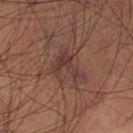Captured during whole-body skin photography for melanoma surveillance; the lesion was not biopsied. The lesion is on the left lower leg. A close-up tile cropped from a whole-body skin photograph, about 15 mm across. A male subject aged 58 to 62. The tile uses cross-polarized illumination. Approximately 4 mm at its widest.